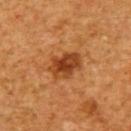This lesion was catalogued during total-body skin photography and was not selected for biopsy.